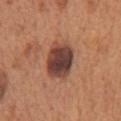Clinical impression: Part of a total-body skin-imaging series; this lesion was reviewed on a skin check and was not flagged for biopsy. Acquisition and patient details: Automated tile analysis of the lesion measured an area of roughly 13 mm² and two-axis asymmetry of about 0.1. And it measured an average lesion color of about L≈41 a*≈22 b*≈26 (CIELAB), a lesion–skin lightness drop of about 16, and a lesion-to-skin contrast of about 13 (normalized; higher = more distinct). Located on the chest. Approximately 4.5 mm at its widest. A 15 mm close-up extracted from a 3D total-body photography capture. A male subject aged approximately 65. Imaged with white-light lighting.